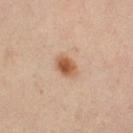Impression:
Captured during whole-body skin photography for melanoma surveillance; the lesion was not biopsied.